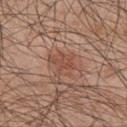The lesion was photographed on a routine skin check and not biopsied; there is no pathology result. A roughly 15 mm field-of-view crop from a total-body skin photograph. The patient is a male about 45 years old. The tile uses white-light illumination. Longest diameter approximately 3.5 mm. Automated tile analysis of the lesion measured border irregularity of about 4.5 on a 0–10 scale, a color-variation rating of about 2.5/10, and peripheral color asymmetry of about 1. It also reported a nevus-likeness score of about 10/100 and a lesion-detection confidence of about 100/100. The lesion is on the upper back.